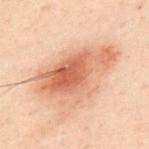biopsy status: imaged on a skin check; not biopsied | imaging modality: ~15 mm tile from a whole-body skin photo | site: the upper back | illumination: cross-polarized illumination | patient: male, roughly 50 years of age.